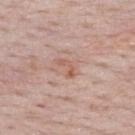Q: Is there a histopathology result?
A: total-body-photography surveillance lesion; no biopsy
Q: Patient demographics?
A: female, aged around 50
Q: How large is the lesion?
A: ~2.5 mm (longest diameter)
Q: What kind of image is this?
A: ~15 mm tile from a whole-body skin photo
Q: What did automated image analysis measure?
A: a lesion area of about 2.5 mm², an outline eccentricity of about 0.9 (0 = round, 1 = elongated), and two-axis asymmetry of about 0.5; a mean CIELAB color near L≈58 a*≈22 b*≈28, roughly 8 lightness units darker than nearby skin, and a normalized lesion–skin contrast near 6; a color-variation rating of about 0/10 and peripheral color asymmetry of about 0; lesion-presence confidence of about 100/100
Q: Lesion location?
A: the back